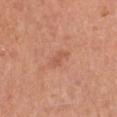The lesion was photographed on a routine skin check and not biopsied; there is no pathology result.
An algorithmic analysis of the crop reported a footprint of about 2.5 mm² and a shape eccentricity near 0.9. The software also gave a lesion color around L≈56 a*≈25 b*≈33 in CIELAB and roughly 7 lightness units darker than nearby skin.
A female patient, aged 58 to 62.
Cropped from a whole-body photographic skin survey; the tile spans about 15 mm.
Captured under white-light illumination.
From the chest.